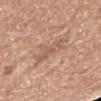Impression:
Imaged during a routine full-body skin examination; the lesion was not biopsied and no histopathology is available.
Context:
From the right upper arm. Measured at roughly 4.5 mm in maximum diameter. The subject is a female roughly 70 years of age. A region of skin cropped from a whole-body photographic capture, roughly 15 mm wide.